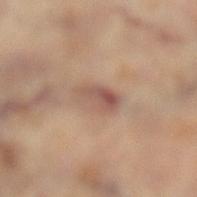Imaged during a routine full-body skin examination; the lesion was not biopsied and no histopathology is available. On the leg. An algorithmic analysis of the crop reported a within-lesion color-variation index near 7/10 and peripheral color asymmetry of about 2.5. A region of skin cropped from a whole-body photographic capture, roughly 15 mm wide. Captured under cross-polarized illumination. The recorded lesion diameter is about 4 mm. A female patient aged 58–62.